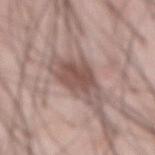Case summary:
* workup: imaged on a skin check; not biopsied
* image source: ~15 mm tile from a whole-body skin photo
* image-analysis metrics: a footprint of about 12 mm², a shape eccentricity near 0.9, and a shape-asymmetry score of about 0.3 (0 = symmetric); a lesion-to-skin contrast of about 9 (normalized; higher = more distinct); internal color variation of about 3 on a 0–10 scale and peripheral color asymmetry of about 1.5; a nevus-likeness score of about 85/100 and a lesion-detection confidence of about 100/100
* site: the front of the torso
* illumination: white-light
* patient: male, aged 58 to 62
* size: ~6 mm (longest diameter)